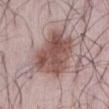workup: catalogued during a skin exam; not biopsied | subject: male, in their 40s | automated lesion analysis: a footprint of about 24 mm², a shape eccentricity near 0.75, and a shape-asymmetry score of about 0.2 (0 = symmetric); a lesion–skin lightness drop of about 13 and a lesion-to-skin contrast of about 9.5 (normalized; higher = more distinct); a border-irregularity rating of about 2.5/10 and a within-lesion color-variation index near 5/10; a nevus-likeness score of about 75/100 and a lesion-detection confidence of about 100/100 | location: the front of the torso | illumination: white-light | imaging modality: total-body-photography crop, ~15 mm field of view.